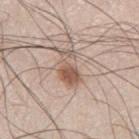follow-up: imaged on a skin check; not biopsied
imaging modality: ~15 mm crop, total-body skin-cancer survey
patient: male, about 60 years old
body site: the left thigh
lesion diameter: about 4.5 mm
tile lighting: white-light
TBP lesion metrics: an area of roughly 8 mm², a shape eccentricity near 0.85, and a shape-asymmetry score of about 0.35 (0 = symmetric)